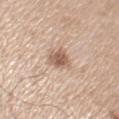Background:
A male subject roughly 70 years of age. Measured at roughly 3.5 mm in maximum diameter. A lesion tile, about 15 mm wide, cut from a 3D total-body photograph. On the left upper arm. This is a white-light tile.